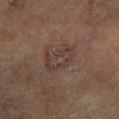An algorithmic analysis of the crop reported a footprint of about 8.5 mm², an eccentricity of roughly 0.8, and a symmetry-axis asymmetry near 0.4. From the right lower leg. Imaged with cross-polarized lighting. A 15 mm crop from a total-body photograph taken for skin-cancer surveillance. Measured at roughly 4 mm in maximum diameter. The subject is a male roughly 65 years of age.